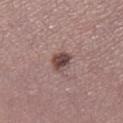<record>
<biopsy_status>not biopsied; imaged during a skin examination</biopsy_status>
<lesion_size>
  <long_diameter_mm_approx>2.5</long_diameter_mm_approx>
</lesion_size>
<patient>
  <sex>female</sex>
  <age_approx>55</age_approx>
</patient>
<site>right lower leg</site>
<image>
  <source>total-body photography crop</source>
  <field_of_view_mm>15</field_of_view_mm>
</image>
<lighting>white-light</lighting>
</record>